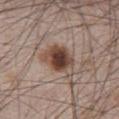- notes · catalogued during a skin exam; not biopsied